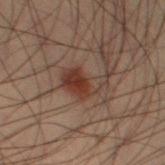Findings:
* TBP lesion metrics: roughly 8 lightness units darker than nearby skin and a lesion-to-skin contrast of about 8.5 (normalized; higher = more distinct)
* lesion diameter: ≈5 mm
* illumination: cross-polarized
* body site: the left thigh
* acquisition: total-body-photography crop, ~15 mm field of view
* subject: male, aged 53–57